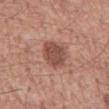automated lesion analysis = a border-irregularity rating of about 2/10
patient = male, in their mid-50s
anatomic site = the mid back
illumination = white-light
diameter = ~4.5 mm (longest diameter)
image = 15 mm crop, total-body photography